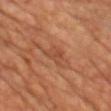• workup · catalogued during a skin exam; not biopsied
• body site · the chest
• TBP lesion metrics · an area of roughly 4.5 mm² and two-axis asymmetry of about 0.45; an average lesion color of about L≈46 a*≈25 b*≈33 (CIELAB) and a normalized lesion–skin contrast near 5; a color-variation rating of about 2.5/10 and a peripheral color-asymmetry measure near 1
• patient · male, approximately 65 years of age
• lesion size · about 3 mm
• image source · 15 mm crop, total-body photography
• illumination · cross-polarized illumination